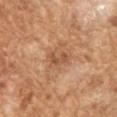Q: Was a biopsy performed?
A: no biopsy performed (imaged during a skin exam)
Q: What is the imaging modality?
A: total-body-photography crop, ~15 mm field of view
Q: Patient demographics?
A: male, roughly 65 years of age